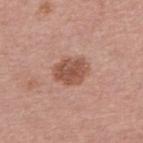Clinical impression: The lesion was tiled from a total-body skin photograph and was not biopsied. Context: Imaged with white-light lighting. A 15 mm close-up extracted from a 3D total-body photography capture. A female subject aged around 50. Automated image analysis of the tile measured an average lesion color of about L≈52 a*≈23 b*≈28 (CIELAB), about 12 CIELAB-L* units darker than the surrounding skin, and a normalized border contrast of about 8. The analysis additionally found a border-irregularity index near 1.5/10 and a peripheral color-asymmetry measure near 1. And it measured a nevus-likeness score of about 30/100 and a lesion-detection confidence of about 100/100. The lesion is on the arm. The lesion's longest dimension is about 4 mm.